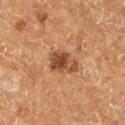Captured during whole-body skin photography for melanoma surveillance; the lesion was not biopsied. A lesion tile, about 15 mm wide, cut from a 3D total-body photograph. The tile uses cross-polarized illumination. Located on the left lower leg. About 3.5 mm across. A male patient, aged around 75.